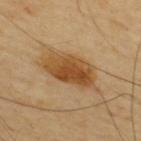- notes: catalogued during a skin exam; not biopsied
- subject: male, in their mid- to late 60s
- site: the upper back
- image source: 15 mm crop, total-body photography